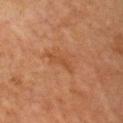Q: Was a biopsy performed?
A: catalogued during a skin exam; not biopsied
Q: Illumination type?
A: cross-polarized illumination
Q: What are the patient's age and sex?
A: male, aged 58 to 62
Q: What kind of image is this?
A: 15 mm crop, total-body photography
Q: How large is the lesion?
A: ~3.5 mm (longest diameter)
Q: Lesion location?
A: the right arm